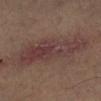The lesion was tiled from a total-body skin photograph and was not biopsied.